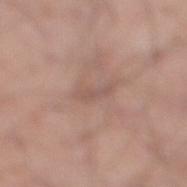notes = imaged on a skin check; not biopsied
body site = the right lower leg
subject = male, aged 38–42
imaging modality = ~15 mm crop, total-body skin-cancer survey
automated metrics = an eccentricity of roughly 0.95; an automated nevus-likeness rating near 0 out of 100 and a lesion-detection confidence of about 65/100
tile lighting = white-light illumination